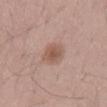<lesion>
  <biopsy_status>not biopsied; imaged during a skin examination</biopsy_status>
  <site>mid back</site>
  <lighting>white-light</lighting>
  <patient>
    <sex>male</sex>
    <age_approx>40</age_approx>
  </patient>
  <automated_metrics>
    <eccentricity>0.55</eccentricity>
    <border_irregularity_0_10>1.5</border_irregularity_0_10>
    <color_variation_0_10>3.0</color_variation_0_10>
    <lesion_detection_confidence_0_100>100</lesion_detection_confidence_0_100>
  </automated_metrics>
  <image>
    <source>total-body photography crop</source>
    <field_of_view_mm>15</field_of_view_mm>
  </image>
  <lesion_size>
    <long_diameter_mm_approx>3.0</long_diameter_mm_approx>
  </lesion_size>
</lesion>